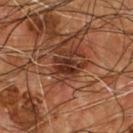No biopsy was performed on this lesion — it was imaged during a full skin examination and was not determined to be concerning. A 15 mm crop from a total-body photograph taken for skin-cancer surveillance. From the chest. A male subject, in their mid- to late 50s.